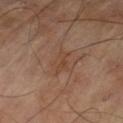Assessment: Imaged during a routine full-body skin examination; the lesion was not biopsied and no histopathology is available. Clinical summary: The tile uses cross-polarized illumination. A male subject, aged 68–72. Located on the left thigh. A 15 mm close-up extracted from a 3D total-body photography capture. Automated image analysis of the tile measured a footprint of about 3.5 mm² and an eccentricity of roughly 0.9. The recorded lesion diameter is about 3 mm.